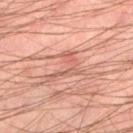Q: Was a biopsy performed?
A: imaged on a skin check; not biopsied
Q: What are the patient's age and sex?
A: male, aged 43–47
Q: How was the tile lit?
A: cross-polarized illumination
Q: Lesion location?
A: the left thigh
Q: How was this image acquired?
A: ~15 mm crop, total-body skin-cancer survey
Q: What is the lesion's diameter?
A: ≈4.5 mm